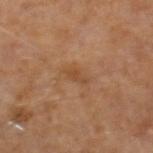Q: Is there a histopathology result?
A: catalogued during a skin exam; not biopsied
Q: How was this image acquired?
A: total-body-photography crop, ~15 mm field of view
Q: What are the patient's age and sex?
A: male, in their 60s
Q: What is the anatomic site?
A: the right lower leg